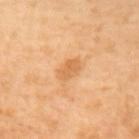{"biopsy_status": "not biopsied; imaged during a skin examination"}